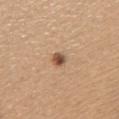<record>
  <biopsy_status>not biopsied; imaged during a skin examination</biopsy_status>
  <lighting>white-light</lighting>
  <image>
    <source>total-body photography crop</source>
    <field_of_view_mm>15</field_of_view_mm>
  </image>
  <patient>
    <sex>female</sex>
    <age_approx>60</age_approx>
  </patient>
  <site>left upper arm</site>
</record>